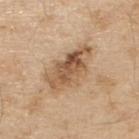workup: no biopsy performed (imaged during a skin exam); tile lighting: white-light illumination; acquisition: ~15 mm tile from a whole-body skin photo; anatomic site: the upper back; subject: male, approximately 70 years of age; lesion size: ≈6.5 mm.